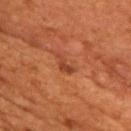The lesion was photographed on a routine skin check and not biopsied; there is no pathology result. A region of skin cropped from a whole-body photographic capture, roughly 15 mm wide. The lesion is on the front of the torso. A male subject, in their mid- to late 50s. Approximately 3 mm at its widest.